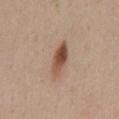Notes:
- lighting — white-light illumination
- image — 15 mm crop, total-body photography
- subject — female, in their 30s
- TBP lesion metrics — border irregularity of about 3 on a 0–10 scale
- anatomic site — the mid back